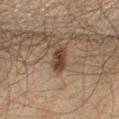biopsy_status: not biopsied; imaged during a skin examination
image:
  source: total-body photography crop
  field_of_view_mm: 15
lighting: cross-polarized
lesion_size:
  long_diameter_mm_approx: 3.5
automated_metrics:
  area_mm2_approx: 5.0
  eccentricity: 0.85
  shape_asymmetry: 0.15
  cielab_L: 30
  cielab_a: 14
  cielab_b: 22
  vs_skin_darker_L: 10.0
  vs_skin_contrast_norm: 10.0
  nevus_likeness_0_100: 95
patient:
  sex: male
  age_approx: 60
site: right thigh